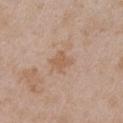automated_metrics:
  vs_skin_darker_L: 7.0
  nevus_likeness_0_100: 0
  lesion_detection_confidence_0_100: 100
lesion_size:
  long_diameter_mm_approx: 2.5
site: chest
lighting: white-light
patient:
  sex: female
  age_approx: 30
image:
  source: total-body photography crop
  field_of_view_mm: 15